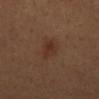Notes:
– workup: imaged on a skin check; not biopsied
– location: the back
– automated metrics: about 6 CIELAB-L* units darker than the surrounding skin and a normalized border contrast of about 6; a border-irregularity index near 3/10 and radial color variation of about 1; a classifier nevus-likeness of about 75/100 and a detector confidence of about 100 out of 100 that the crop contains a lesion
– lesion diameter: about 3.5 mm
– image: ~15 mm crop, total-body skin-cancer survey
– patient: male, aged 63–67